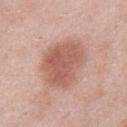  site: front of the torso
  automated_metrics:
    area_mm2_approx: 22.0
    shape_asymmetry: 0.2
  lesion_size:
    long_diameter_mm_approx: 6.5
  patient:
    sex: male
    age_approx: 55
  image:
    source: total-body photography crop
    field_of_view_mm: 15